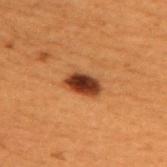Assessment:
Imaged during a routine full-body skin examination; the lesion was not biopsied and no histopathology is available.
Context:
The lesion is located on the upper back. Cropped from a total-body skin-imaging series; the visible field is about 15 mm. The patient is a male aged 48 to 52.